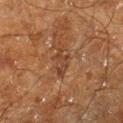Acquisition and patient details: From the right lower leg. Cropped from a total-body skin-imaging series; the visible field is about 15 mm. An algorithmic analysis of the crop reported a footprint of about 5 mm², an eccentricity of roughly 0.9, and a symmetry-axis asymmetry near 0.25. The analysis additionally found a lesion color around L≈31 a*≈18 b*≈26 in CIELAB, a lesion–skin lightness drop of about 6, and a normalized border contrast of about 6.5. The software also gave a border-irregularity index near 4.5/10, internal color variation of about 1 on a 0–10 scale, and peripheral color asymmetry of about 0.5. The analysis additionally found a lesion-detection confidence of about 60/100. Imaged with cross-polarized lighting. A male subject, aged around 60. The lesion's longest dimension is about 4 mm.